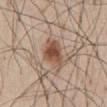| feature | finding |
|---|---|
| biopsy status | catalogued during a skin exam; not biopsied |
| automated metrics | a footprint of about 9 mm², an outline eccentricity of about 0.65 (0 = round, 1 = elongated), and two-axis asymmetry of about 0.25; a mean CIELAB color near L≈51 a*≈19 b*≈28, roughly 12 lightness units darker than nearby skin, and a lesion-to-skin contrast of about 9 (normalized; higher = more distinct); a border-irregularity index near 2.5/10 and a within-lesion color-variation index near 5/10 |
| lesion diameter | about 4 mm |
| tile lighting | white-light illumination |
| acquisition | ~15 mm tile from a whole-body skin photo |
| subject | male, about 60 years old |
| location | the abdomen |